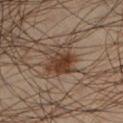This lesion was catalogued during total-body skin photography and was not selected for biopsy.
About 4 mm across.
On the left lower leg.
An algorithmic analysis of the crop reported a shape eccentricity near 0.5 and a shape-asymmetry score of about 0.35 (0 = symmetric).
A male subject, aged approximately 45.
This is a cross-polarized tile.
A 15 mm crop from a total-body photograph taken for skin-cancer surveillance.